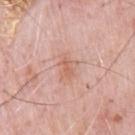The lesion was photographed on a routine skin check and not biopsied; there is no pathology result. A male patient, aged approximately 80. Located on the chest. Cropped from a total-body skin-imaging series; the visible field is about 15 mm. Automated tile analysis of the lesion measured a lesion color around L≈63 a*≈23 b*≈30 in CIELAB and about 7 CIELAB-L* units darker than the surrounding skin. The software also gave a color-variation rating of about 2.5/10 and peripheral color asymmetry of about 1. It also reported an automated nevus-likeness rating near 0 out of 100 and a lesion-detection confidence of about 100/100. Captured under white-light illumination. The recorded lesion diameter is about 2.5 mm.